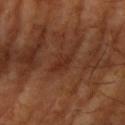Imaged during a routine full-body skin examination; the lesion was not biopsied and no histopathology is available. The lesion is on the right upper arm. The tile uses cross-polarized illumination. A male subject, aged 63–67. Automated image analysis of the tile measured a lesion color around L≈30 a*≈23 b*≈29 in CIELAB, roughly 6 lightness units darker than nearby skin, and a normalized border contrast of about 6. It also reported a border-irregularity index near 4.5/10, a within-lesion color-variation index near 1/10, and a peripheral color-asymmetry measure near 0.5. A 15 mm close-up tile from a total-body photography series done for melanoma screening. Measured at roughly 4 mm in maximum diameter.